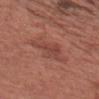biopsy status: no biopsy performed (imaged during a skin exam).